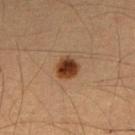follow-up — no biopsy performed (imaged during a skin exam) | location — the right forearm | subject — male, aged approximately 35 | imaging modality — total-body-photography crop, ~15 mm field of view | lighting — cross-polarized illumination | diameter — about 2.5 mm.